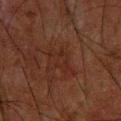Part of a total-body skin-imaging series; this lesion was reviewed on a skin check and was not flagged for biopsy. Cropped from a total-body skin-imaging series; the visible field is about 15 mm. Imaged with cross-polarized lighting. Located on the upper back. The patient is a male in their mid-60s. Longest diameter approximately 4 mm.